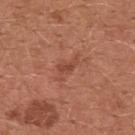Impression: No biopsy was performed on this lesion — it was imaged during a full skin examination and was not determined to be concerning. Context: A roughly 15 mm field-of-view crop from a total-body skin photograph. Longest diameter approximately 3.5 mm. An algorithmic analysis of the crop reported two-axis asymmetry of about 0.55. And it measured a mean CIELAB color near L≈47 a*≈26 b*≈31, about 7 CIELAB-L* units darker than the surrounding skin, and a lesion-to-skin contrast of about 5.5 (normalized; higher = more distinct). The analysis additionally found a border-irregularity rating of about 6.5/10 and peripheral color asymmetry of about 0.5. It also reported a classifier nevus-likeness of about 0/100. The lesion is located on the chest. A female patient, roughly 35 years of age.